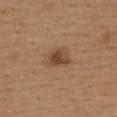biopsy_status: not biopsied; imaged during a skin examination
patient:
  sex: female
  age_approx: 40
image:
  source: total-body photography crop
  field_of_view_mm: 15
lighting: white-light
site: back